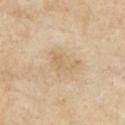Notes:
• notes · no biopsy performed (imaged during a skin exam)
• image-analysis metrics · a lesion color around L≈67 a*≈14 b*≈37 in CIELAB; a border-irregularity rating of about 4.5/10 and peripheral color asymmetry of about 1
• lighting · cross-polarized illumination
• image · total-body-photography crop, ~15 mm field of view
• patient · female, aged approximately 70
• body site · the chest
• lesion diameter · about 4 mm